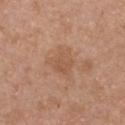{
  "biopsy_status": "not biopsied; imaged during a skin examination",
  "image": {
    "source": "total-body photography crop",
    "field_of_view_mm": 15
  },
  "lesion_size": {
    "long_diameter_mm_approx": 4.0
  },
  "lighting": "white-light",
  "patient": {
    "sex": "female",
    "age_approx": 30
  },
  "site": "chest"
}